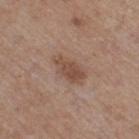Part of a total-body skin-imaging series; this lesion was reviewed on a skin check and was not flagged for biopsy. The lesion is on the right thigh. Cropped from a whole-body photographic skin survey; the tile spans about 15 mm. Measured at roughly 4 mm in maximum diameter. A female subject aged around 45.